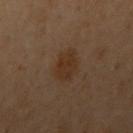No biopsy was performed on this lesion — it was imaged during a full skin examination and was not determined to be concerning. The subject is a female approximately 60 years of age. A 15 mm crop from a total-body photograph taken for skin-cancer surveillance. Imaged with cross-polarized lighting. Longest diameter approximately 4 mm. The lesion is on the chest.